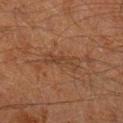{
  "biopsy_status": "not biopsied; imaged during a skin examination",
  "patient": {
    "sex": "male",
    "age_approx": 60
  },
  "image": {
    "source": "total-body photography crop",
    "field_of_view_mm": 15
  },
  "lighting": "cross-polarized",
  "site": "right lower leg"
}